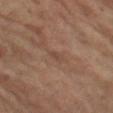Captured during whole-body skin photography for melanoma surveillance; the lesion was not biopsied. The lesion is on the leg. The lesion-visualizer software estimated a lesion area of about 2 mm² and a shape eccentricity near 0.95. And it measured an average lesion color of about L≈43 a*≈18 b*≈26 (CIELAB), a lesion–skin lightness drop of about 6, and a lesion-to-skin contrast of about 5 (normalized; higher = more distinct). And it measured border irregularity of about 3.5 on a 0–10 scale, internal color variation of about 0 on a 0–10 scale, and a peripheral color-asymmetry measure near 0. And it measured a nevus-likeness score of about 0/100 and a detector confidence of about 55 out of 100 that the crop contains a lesion. A roughly 15 mm field-of-view crop from a total-body skin photograph. A female subject, aged 68 to 72. About 2.5 mm across.